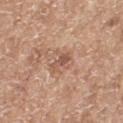This lesion was catalogued during total-body skin photography and was not selected for biopsy.
The lesion is on the left thigh.
A roughly 15 mm field-of-view crop from a total-body skin photograph.
The lesion-visualizer software estimated a lesion area of about 5.5 mm² and a shape eccentricity near 0.65. It also reported roughly 9 lightness units darker than nearby skin. It also reported a border-irregularity rating of about 4.5/10, internal color variation of about 3.5 on a 0–10 scale, and peripheral color asymmetry of about 1.
A female subject in their mid-70s.